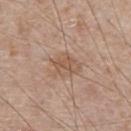Findings:
• workup · catalogued during a skin exam; not biopsied
• size · about 4 mm
• image source · ~15 mm tile from a whole-body skin photo
• body site · the abdomen
• lighting · white-light illumination
• TBP lesion metrics · an area of roughly 8.5 mm² and an outline eccentricity of about 0.65 (0 = round, 1 = elongated); a mean CIELAB color near L≈55 a*≈18 b*≈29, roughly 8 lightness units darker than nearby skin, and a normalized lesion–skin contrast near 6; border irregularity of about 3 on a 0–10 scale
• subject · male, approximately 65 years of age